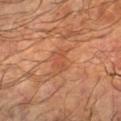follow-up: imaged on a skin check; not biopsied | anatomic site: the left forearm | image-analysis metrics: a lesion area of about 3 mm² and an outline eccentricity of about 0.85 (0 = round, 1 = elongated); border irregularity of about 5 on a 0–10 scale and a peripheral color-asymmetry measure near 0; a lesion-detection confidence of about 100/100 | diameter: ≈3 mm | patient: male, in their 60s | imaging modality: 15 mm crop, total-body photography | tile lighting: cross-polarized.